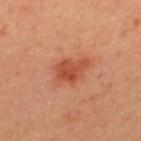<record>
  <biopsy_status>not biopsied; imaged during a skin examination</biopsy_status>
  <site>back</site>
  <lighting>cross-polarized</lighting>
  <patient>
    <sex>female</sex>
    <age_approx>45</age_approx>
  </patient>
  <automated_metrics>
    <eccentricity>0.75</eccentricity>
    <shape_asymmetry>0.35</shape_asymmetry>
    <border_irregularity_0_10>3.5</border_irregularity_0_10>
    <peripheral_color_asymmetry>1.0</peripheral_color_asymmetry>
    <nevus_likeness_0_100>100</nevus_likeness_0_100>
  </automated_metrics>
  <image>
    <source>total-body photography crop</source>
    <field_of_view_mm>15</field_of_view_mm>
  </image>
  <lesion_size>
    <long_diameter_mm_approx>3.5</long_diameter_mm_approx>
  </lesion_size>
</record>